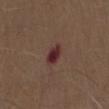biopsy status — imaged on a skin check; not biopsied
body site — the chest
illumination — white-light illumination
TBP lesion metrics — a lesion area of about 5 mm², a shape eccentricity near 0.8, and a shape-asymmetry score of about 0.2 (0 = symmetric); an average lesion color of about L≈31 a*≈22 b*≈19 (CIELAB), a lesion–skin lightness drop of about 11, and a normalized border contrast of about 10.5; a border-irregularity index near 2/10 and radial color variation of about 1; a classifier nevus-likeness of about 0/100 and a lesion-detection confidence of about 100/100
imaging modality — ~15 mm tile from a whole-body skin photo
patient — male, aged approximately 70